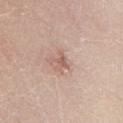Assessment:
Recorded during total-body skin imaging; not selected for excision or biopsy.
Image and clinical context:
The lesion is on the right lower leg. A 15 mm close-up extracted from a 3D total-body photography capture. A female patient aged approximately 70. Imaged with white-light lighting. Automated image analysis of the tile measured a footprint of about 2 mm², an eccentricity of roughly 0.7, and two-axis asymmetry of about 0.35. The analysis additionally found a border-irregularity rating of about 3.5/10 and internal color variation of about 0 on a 0–10 scale. It also reported a nevus-likeness score of about 0/100 and lesion-presence confidence of about 100/100. Approximately 2 mm at its widest.